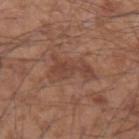follow-up: imaged on a skin check; not biopsied
site: the left forearm
automated metrics: a shape eccentricity near 0.8 and two-axis asymmetry of about 0.45; an average lesion color of about L≈44 a*≈21 b*≈27 (CIELAB), roughly 7 lightness units darker than nearby skin, and a normalized border contrast of about 5.5; a color-variation rating of about 3/10 and peripheral color asymmetry of about 1
lighting: white-light illumination
patient: male, aged 53 to 57
acquisition: ~15 mm tile from a whole-body skin photo
diameter: about 5 mm